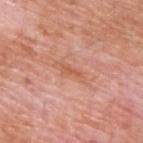The tile uses white-light illumination.
A male subject, approximately 60 years of age.
The lesion is located on the upper back.
The total-body-photography lesion software estimated an area of roughly 2 mm² and two-axis asymmetry of about 0.35. The software also gave a border-irregularity index near 4.5/10 and internal color variation of about 0 on a 0–10 scale. The software also gave a classifier nevus-likeness of about 0/100 and lesion-presence confidence of about 95/100.
Cropped from a whole-body photographic skin survey; the tile spans about 15 mm.
Measured at roughly 2.5 mm in maximum diameter.